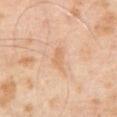Notes:
- biopsy status — no biopsy performed (imaged during a skin exam)
- lesion size — ≈3 mm
- imaging modality — ~15 mm tile from a whole-body skin photo
- tile lighting — cross-polarized illumination
- patient — male, aged 63 to 67